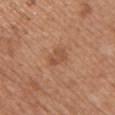workup: catalogued during a skin exam; not biopsied | image-analysis metrics: an area of roughly 4.5 mm² and an outline eccentricity of about 0.7 (0 = round, 1 = elongated); a within-lesion color-variation index near 2.5/10 and radial color variation of about 1 | lighting: white-light illumination | image: ~15 mm crop, total-body skin-cancer survey | subject: female, roughly 55 years of age | anatomic site: the arm | diameter: ~2.5 mm (longest diameter).